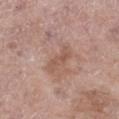notes=total-body-photography surveillance lesion; no biopsy | diameter=≈3.5 mm | automated metrics=a border-irregularity rating of about 4.5/10, internal color variation of about 0.5 on a 0–10 scale, and radial color variation of about 0 | image source=15 mm crop, total-body photography | site=the right lower leg | lighting=white-light illumination | patient=female, aged around 75.